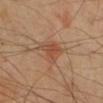<record>
<image>
  <source>total-body photography crop</source>
  <field_of_view_mm>15</field_of_view_mm>
</image>
<patient>
  <sex>male</sex>
  <age_approx>70</age_approx>
</patient>
<lesion_size>
  <long_diameter_mm_approx>4.0</long_diameter_mm_approx>
</lesion_size>
<lighting>cross-polarized</lighting>
<site>right lower leg</site>
</record>